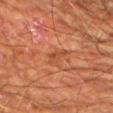Imaged during a routine full-body skin examination; the lesion was not biopsied and no histopathology is available. This is a cross-polarized tile. Approximately 3.5 mm at its widest. The lesion is on the left upper arm. A male subject, about 65 years old. A region of skin cropped from a whole-body photographic capture, roughly 15 mm wide.